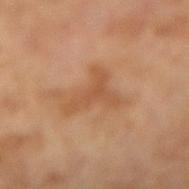Imaged during a routine full-body skin examination; the lesion was not biopsied and no histopathology is available. A female subject, aged 68 to 72. The tile uses cross-polarized illumination. On the right lower leg. Longest diameter approximately 5 mm. A roughly 15 mm field-of-view crop from a total-body skin photograph.